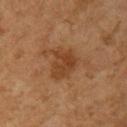notes: imaged on a skin check; not biopsied
lesion diameter: ~3.5 mm (longest diameter)
site: the left upper arm
patient: female, aged approximately 60
image: 15 mm crop, total-body photography
image-analysis metrics: an area of roughly 9.5 mm²; a nevus-likeness score of about 25/100 and lesion-presence confidence of about 100/100
lighting: cross-polarized